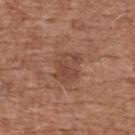Case summary:
– workup · total-body-photography surveillance lesion; no biopsy
– subject · male, about 75 years old
– imaging modality · 15 mm crop, total-body photography
– tile lighting · white-light illumination
– site · the upper back
– automated lesion analysis · a footprint of about 9 mm² and a shape eccentricity near 0.6; an average lesion color of about L≈45 a*≈22 b*≈28 (CIELAB) and a lesion–skin lightness drop of about 7; a lesion-detection confidence of about 100/100